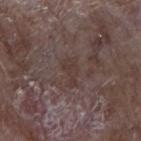Clinical impression:
The lesion was tiled from a total-body skin photograph and was not biopsied.